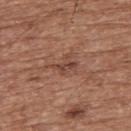Recorded during total-body skin imaging; not selected for excision or biopsy.
A male subject, approximately 75 years of age.
The lesion is on the upper back.
This image is a 15 mm lesion crop taken from a total-body photograph.
This is a white-light tile.
Longest diameter approximately 3 mm.